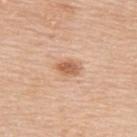TBP lesion metrics: a lesion color around L≈62 a*≈22 b*≈34 in CIELAB, about 11 CIELAB-L* units darker than the surrounding skin, and a lesion-to-skin contrast of about 7.5 (normalized; higher = more distinct); a within-lesion color-variation index near 2.5/10 and a peripheral color-asymmetry measure near 1; an automated nevus-likeness rating near 90 out of 100
lesion diameter: about 3 mm
anatomic site: the upper back
tile lighting: white-light illumination
image: 15 mm crop, total-body photography
subject: male, aged approximately 80